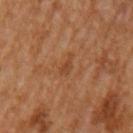<tbp_lesion>
<biopsy_status>not biopsied; imaged during a skin examination</biopsy_status>
<site>left upper arm</site>
<image>
  <source>total-body photography crop</source>
  <field_of_view_mm>15</field_of_view_mm>
</image>
<patient>
  <sex>male</sex>
  <age_approx>65</age_approx>
</patient>
</tbp_lesion>